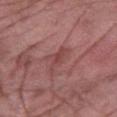Case summary:
* biopsy status · catalogued during a skin exam; not biopsied
* tile lighting · white-light
* automated lesion analysis · a mean CIELAB color near L≈45 a*≈25 b*≈22 and a lesion-to-skin contrast of about 5.5 (normalized; higher = more distinct)
* lesion diameter · about 4 mm
* subject · male, aged approximately 55
* image source · ~15 mm tile from a whole-body skin photo
* location · the right forearm